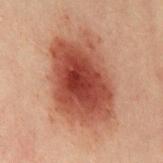Part of a total-body skin-imaging series; this lesion was reviewed on a skin check and was not flagged for biopsy. A 15 mm close-up extracted from a 3D total-body photography capture. An algorithmic analysis of the crop reported about 13 CIELAB-L* units darker than the surrounding skin. The software also gave a classifier nevus-likeness of about 100/100 and a detector confidence of about 100 out of 100 that the crop contains a lesion. Approximately 8.5 mm at its widest. The patient is a female aged approximately 20. The lesion is located on the chest.